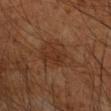patient: male, aged around 60
site: the left forearm
lighting: cross-polarized
image source: ~15 mm tile from a whole-body skin photo